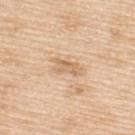No biopsy was performed on this lesion — it was imaged during a full skin examination and was not determined to be concerning.
The lesion is on the upper back.
A female patient, about 55 years old.
This image is a 15 mm lesion crop taken from a total-body photograph.